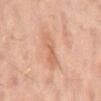The lesion was photographed on a routine skin check and not biopsied; there is no pathology result. Cropped from a whole-body photographic skin survey; the tile spans about 15 mm. From the mid back. The subject is a male roughly 60 years of age.